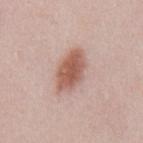Clinical impression: The lesion was tiled from a total-body skin photograph and was not biopsied. Background: Imaged with white-light lighting. Cropped from a total-body skin-imaging series; the visible field is about 15 mm. Located on the back. A male patient, in their mid-20s. Longest diameter approximately 5.5 mm.